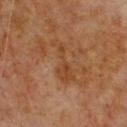workup=no biopsy performed (imaged during a skin exam); patient=male, aged 78 to 82; acquisition=total-body-photography crop, ~15 mm field of view; body site=the chest.